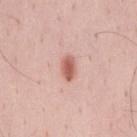automated metrics: a lesion area of about 4 mm², an outline eccentricity of about 0.8 (0 = round, 1 = elongated), and a symmetry-axis asymmetry near 0.2; an average lesion color of about L≈60 a*≈26 b*≈28 (CIELAB) and a normalized lesion–skin contrast near 9
anatomic site: the back
lesion size: about 3 mm
image: total-body-photography crop, ~15 mm field of view
subject: male, aged 33 to 37